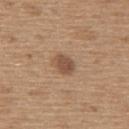<case>
  <biopsy_status>not biopsied; imaged during a skin examination</biopsy_status>
  <automated_metrics>
    <area_mm2_approx>5.0</area_mm2_approx>
    <eccentricity>0.7</eccentricity>
    <shape_asymmetry>0.15</shape_asymmetry>
    <cielab_L>50</cielab_L>
    <cielab_a>19</cielab_a>
    <cielab_b>30</cielab_b>
    <vs_skin_darker_L>11.0</vs_skin_darker_L>
    <lesion_detection_confidence_0_100>100</lesion_detection_confidence_0_100>
  </automated_metrics>
  <lighting>white-light</lighting>
  <image>
    <source>total-body photography crop</source>
    <field_of_view_mm>15</field_of_view_mm>
  </image>
  <patient>
    <sex>male</sex>
    <age_approx>65</age_approx>
  </patient>
  <site>upper back</site>
</case>